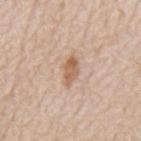<lesion>
  <biopsy_status>not biopsied; imaged during a skin examination</biopsy_status>
  <image>
    <source>total-body photography crop</source>
    <field_of_view_mm>15</field_of_view_mm>
  </image>
  <site>mid back</site>
  <lighting>white-light</lighting>
  <patient>
    <sex>male</sex>
    <age_approx>80</age_approx>
  </patient>
</lesion>